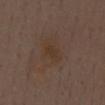{"biopsy_status": "not biopsied; imaged during a skin examination", "patient": {"sex": "male", "age_approx": 70}, "site": "chest", "lesion_size": {"long_diameter_mm_approx": 3.0}, "image": {"source": "total-body photography crop", "field_of_view_mm": 15}, "lighting": "white-light", "automated_metrics": {"cielab_L": 34, "cielab_a": 15, "cielab_b": 26, "vs_skin_darker_L": 4.0, "border_irregularity_0_10": 2.0, "color_variation_0_10": 1.5, "peripheral_color_asymmetry": 0.5, "nevus_likeness_0_100": 0, "lesion_detection_confidence_0_100": 100}}